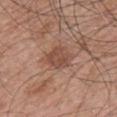biopsy status = total-body-photography surveillance lesion; no biopsy | illumination = white-light | lesion size = about 4 mm | body site = the chest | image source = ~15 mm tile from a whole-body skin photo | subject = male, in their 70s.